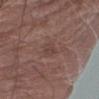follow-up — imaged on a skin check; not biopsied | image-analysis metrics — a border-irregularity rating of about 4/10 and radial color variation of about 0.5 | lighting — white-light | patient — male, aged around 65 | body site — the right forearm | acquisition — 15 mm crop, total-body photography.